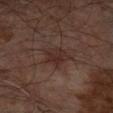Assessment: The lesion was tiled from a total-body skin photograph and was not biopsied. Context: A 15 mm crop from a total-body photograph taken for skin-cancer surveillance. From the left forearm. This is a cross-polarized tile. The subject is a male aged approximately 65. Longest diameter approximately 3.5 mm.